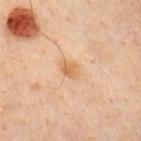Clinical impression: Part of a total-body skin-imaging series; this lesion was reviewed on a skin check and was not flagged for biopsy. Acquisition and patient details: The total-body-photography lesion software estimated a footprint of about 6 mm² and two-axis asymmetry of about 0.2. A male patient, aged around 50. Measured at roughly 3.5 mm in maximum diameter. A 15 mm crop from a total-body photograph taken for skin-cancer surveillance. From the chest. The tile uses cross-polarized illumination.